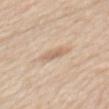workup: catalogued during a skin exam; not biopsied | patient: male, about 60 years old | image source: total-body-photography crop, ~15 mm field of view | illumination: white-light illumination | size: about 3.5 mm | location: the mid back.